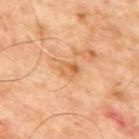Impression:
Recorded during total-body skin imaging; not selected for excision or biopsy.
Background:
Cropped from a whole-body photographic skin survey; the tile spans about 15 mm. The subject is a male roughly 65 years of age. Captured under cross-polarized illumination. The lesion is located on the upper back. Longest diameter approximately 2.5 mm.